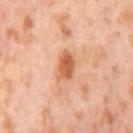Q: Is there a histopathology result?
A: total-body-photography surveillance lesion; no biopsy
Q: Where on the body is the lesion?
A: the right thigh
Q: What kind of image is this?
A: 15 mm crop, total-body photography
Q: What are the patient's age and sex?
A: female, in their mid- to late 50s
Q: Illumination type?
A: cross-polarized
Q: How large is the lesion?
A: ≈3.5 mm